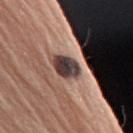workup=no biopsy performed (imaged during a skin exam) | patient=female, aged around 65 | anatomic site=the right upper arm | size=about 3.5 mm | image source=15 mm crop, total-body photography | illumination=white-light illumination.